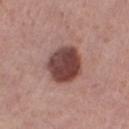Clinical impression: Imaged during a routine full-body skin examination; the lesion was not biopsied and no histopathology is available. Context: The lesion's longest dimension is about 5 mm. A 15 mm close-up tile from a total-body photography series done for melanoma screening. A female patient, aged 63–67. An algorithmic analysis of the crop reported a mean CIELAB color near L≈43 a*≈22 b*≈22, about 17 CIELAB-L* units darker than the surrounding skin, and a normalized border contrast of about 12.5. The software also gave an automated nevus-likeness rating near 40 out of 100 and lesion-presence confidence of about 100/100. The lesion is on the left lower leg. This is a white-light tile.